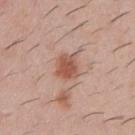biopsy status — imaged on a skin check; not biopsied | size — about 3 mm | site — the chest | patient — male, aged 28–32 | TBP lesion metrics — an average lesion color of about L≈55 a*≈23 b*≈28 (CIELAB), roughly 11 lightness units darker than nearby skin, and a lesion-to-skin contrast of about 8 (normalized; higher = more distinct); a border-irregularity rating of about 2/10, a within-lesion color-variation index near 3/10, and radial color variation of about 1; a nevus-likeness score of about 90/100 | illumination — white-light | acquisition — ~15 mm tile from a whole-body skin photo.